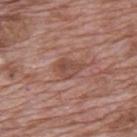No biopsy was performed on this lesion — it was imaged during a full skin examination and was not determined to be concerning. From the back. A male patient, approximately 70 years of age. Approximately 3.5 mm at its widest. This image is a 15 mm lesion crop taken from a total-body photograph. This is a white-light tile. Automated image analysis of the tile measured an area of roughly 6 mm², an eccentricity of roughly 0.75, and two-axis asymmetry of about 0.35. And it measured an automated nevus-likeness rating near 0 out of 100.